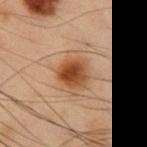biopsy status = catalogued during a skin exam; not biopsied | acquisition = 15 mm crop, total-body photography | site = the chest | subject = male, approximately 55 years of age.